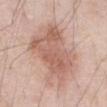The lesion was photographed on a routine skin check and not biopsied; there is no pathology result. Captured under white-light illumination. The patient is a male aged approximately 70. A 15 mm close-up tile from a total-body photography series done for melanoma screening. From the abdomen. The lesion's longest dimension is about 8 mm.